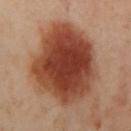Impression:
Part of a total-body skin-imaging series; this lesion was reviewed on a skin check and was not flagged for biopsy.
Context:
The lesion's longest dimension is about 8.5 mm. A close-up tile cropped from a whole-body skin photograph, about 15 mm across. The lesion-visualizer software estimated a lesion area of about 50 mm², an eccentricity of roughly 0.5, and a symmetry-axis asymmetry near 0.15. It also reported a border-irregularity index near 2/10, a within-lesion color-variation index near 6/10, and a peripheral color-asymmetry measure near 1.5. A female subject, about 40 years old. The lesion is on the left arm. Imaged with cross-polarized lighting.